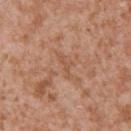<lesion>
<biopsy_status>not biopsied; imaged during a skin examination</biopsy_status>
<lesion_size>
  <long_diameter_mm_approx>3.0</long_diameter_mm_approx>
</lesion_size>
<automated_metrics>
  <area_mm2_approx>3.0</area_mm2_approx>
  <eccentricity>0.9</eccentricity>
  <shape_asymmetry>0.45</shape_asymmetry>
  <cielab_L>54</cielab_L>
  <cielab_a>22</cielab_a>
  <cielab_b>33</cielab_b>
  <vs_skin_darker_L>6.0</vs_skin_darker_L>
  <vs_skin_contrast_norm>4.5</vs_skin_contrast_norm>
  <nevus_likeness_0_100>0</nevus_likeness_0_100>
  <lesion_detection_confidence_0_100>90</lesion_detection_confidence_0_100>
</automated_metrics>
<patient>
  <sex>male</sex>
  <age_approx>45</age_approx>
</patient>
<site>left upper arm</site>
<lighting>white-light</lighting>
<image>
  <source>total-body photography crop</source>
  <field_of_view_mm>15</field_of_view_mm>
</image>
</lesion>